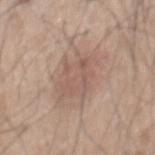Imaged during a routine full-body skin examination; the lesion was not biopsied and no histopathology is available.